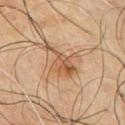{"biopsy_status": "not biopsied; imaged during a skin examination"}